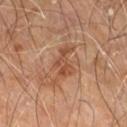Automated image analysis of the tile measured an area of roughly 7.5 mm², an outline eccentricity of about 0.85 (0 = round, 1 = elongated), and a symmetry-axis asymmetry near 0.55. It also reported a lesion color around L≈48 a*≈22 b*≈32 in CIELAB, about 9 CIELAB-L* units darker than the surrounding skin, and a normalized lesion–skin contrast near 6.5. The analysis additionally found border irregularity of about 7.5 on a 0–10 scale, a color-variation rating of about 3/10, and a peripheral color-asymmetry measure near 1. This image is a 15 mm lesion crop taken from a total-body photograph. The subject is a male aged around 60. The lesion's longest dimension is about 5 mm. Imaged with cross-polarized lighting. The lesion is located on the right leg.